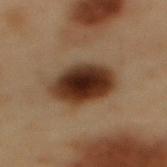The lesion was photographed on a routine skin check and not biopsied; there is no pathology result.